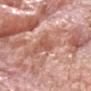{"patient": {"sex": "male", "age_approx": 60}, "site": "head or neck", "image": {"source": "total-body photography crop", "field_of_view_mm": 15}, "diagnosis": {"histopathology": "invasive squamous cell carcinoma", "malignancy": "malignant", "taxonomic_path": ["Malignant", "Malignant epidermal proliferations", "Squamous cell carcinoma, Invasive"]}}